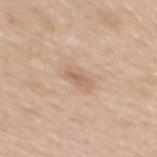Recorded during total-body skin imaging; not selected for excision or biopsy. This image is a 15 mm lesion crop taken from a total-body photograph. About 2.5 mm across. A male patient, approximately 50 years of age. On the back. An algorithmic analysis of the crop reported a lesion color around L≈62 a*≈18 b*≈30 in CIELAB and about 8 CIELAB-L* units darker than the surrounding skin. And it measured a border-irregularity index near 2/10 and a peripheral color-asymmetry measure near 0. This is a white-light tile.